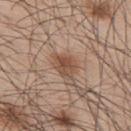Q: Was a biopsy performed?
A: no biopsy performed (imaged during a skin exam)
Q: Lesion size?
A: ≈4 mm
Q: Lesion location?
A: the upper back
Q: How was the tile lit?
A: white-light illumination
Q: Automated lesion metrics?
A: a border-irregularity index near 2.5/10 and internal color variation of about 4 on a 0–10 scale
Q: Who is the patient?
A: male, aged 43 to 47
Q: What kind of image is this?
A: 15 mm crop, total-body photography